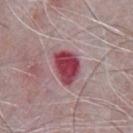biopsy_status: not biopsied; imaged during a skin examination
site: chest
lesion_size:
  long_diameter_mm_approx: 4.0
patient:
  sex: male
  age_approx: 65
lighting: white-light
automated_metrics:
  area_mm2_approx: 9.5
  shape_asymmetry: 0.25
  cielab_L: 43
  cielab_a: 34
  cielab_b: 17
  vs_skin_darker_L: 17.0
  vs_skin_contrast_norm: 12.5
image:
  source: total-body photography crop
  field_of_view_mm: 15